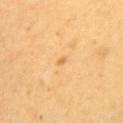Impression:
The lesion was tiled from a total-body skin photograph and was not biopsied.